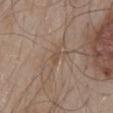biopsy_status: not biopsied; imaged during a skin examination
site: mid back
automated_metrics:
  cielab_L: 49
  cielab_a: 15
  cielab_b: 27
  vs_skin_darker_L: 5.0
patient:
  sex: male
  age_approx: 55
image:
  source: total-body photography crop
  field_of_view_mm: 15
lesion_size:
  long_diameter_mm_approx: 1.0